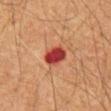Background:
The recorded lesion diameter is about 3 mm. Imaged with cross-polarized lighting. A male patient, approximately 60 years of age. Located on the mid back. The total-body-photography lesion software estimated a lesion area of about 6.5 mm², a shape eccentricity near 0.4, and a shape-asymmetry score of about 0.15 (0 = symmetric). And it measured about 16 CIELAB-L* units darker than the surrounding skin and a normalized border contrast of about 12. It also reported border irregularity of about 1.5 on a 0–10 scale, internal color variation of about 4 on a 0–10 scale, and radial color variation of about 1.5. The analysis additionally found a classifier nevus-likeness of about 0/100 and a detector confidence of about 100 out of 100 that the crop contains a lesion. A region of skin cropped from a whole-body photographic capture, roughly 15 mm wide.